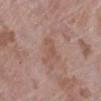Q: Where on the body is the lesion?
A: the left lower leg
Q: Who is the patient?
A: female, about 70 years old
Q: What kind of image is this?
A: total-body-photography crop, ~15 mm field of view